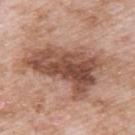Q: Was a biopsy performed?
A: total-body-photography surveillance lesion; no biopsy
Q: How was this image acquired?
A: ~15 mm tile from a whole-body skin photo
Q: What are the patient's age and sex?
A: male, in their 50s
Q: Illumination type?
A: white-light illumination
Q: Where on the body is the lesion?
A: the upper back
Q: How large is the lesion?
A: about 8 mm